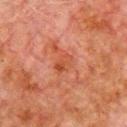Captured under cross-polarized illumination.
A region of skin cropped from a whole-body photographic capture, roughly 15 mm wide.
Approximately 2.5 mm at its widest.
On the chest.
The patient is a male aged approximately 80.